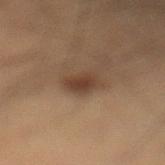Image and clinical context: A male patient, aged approximately 55. The lesion-visualizer software estimated a lesion–skin lightness drop of about 8. The lesion is located on the leg. Cropped from a total-body skin-imaging series; the visible field is about 15 mm. Captured under cross-polarized illumination. The recorded lesion diameter is about 3 mm.